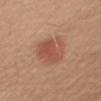Imaged during a routine full-body skin examination; the lesion was not biopsied and no histopathology is available.
The lesion is on the right upper arm.
Longest diameter approximately 4.5 mm.
A 15 mm crop from a total-body photograph taken for skin-cancer surveillance.
An algorithmic analysis of the crop reported a lesion area of about 14 mm², an outline eccentricity of about 0.6 (0 = round, 1 = elongated), and a symmetry-axis asymmetry near 0.15. It also reported an average lesion color of about L≈52 a*≈25 b*≈30 (CIELAB) and a lesion-to-skin contrast of about 6.5 (normalized; higher = more distinct). And it measured a color-variation rating of about 3.5/10 and radial color variation of about 1.
A male subject aged around 30.